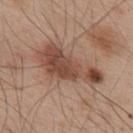follow-up=no biopsy performed (imaged during a skin exam)
patient=male, aged around 55
lighting=white-light
acquisition=15 mm crop, total-body photography
site=the upper back
automated metrics=a color-variation rating of about 6/10 and radial color variation of about 2
lesion diameter=~8 mm (longest diameter)